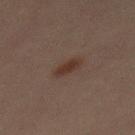biopsy_status: not biopsied; imaged during a skin examination
lesion_size:
  long_diameter_mm_approx: 3.0
site: mid back
image:
  source: total-body photography crop
  field_of_view_mm: 15
patient:
  sex: male
  age_approx: 30